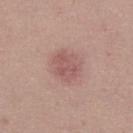  lighting: white-light
  patient:
    sex: female
    age_approx: 20
  image:
    source: total-body photography crop
    field_of_view_mm: 15
  site: right lower leg
  automated_metrics:
    area_mm2_approx: 11.0
    eccentricity: 0.5
    shape_asymmetry: 0.15